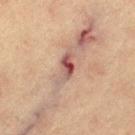notes: catalogued during a skin exam; not biopsied | subject: female, about 65 years old | image: ~15 mm tile from a whole-body skin photo | lesion size: ≈4 mm | anatomic site: the left thigh.